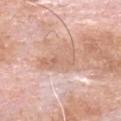Recorded during total-body skin imaging; not selected for excision or biopsy. A male patient, about 80 years old. A roughly 15 mm field-of-view crop from a total-body skin photograph. The lesion is on the head or neck.